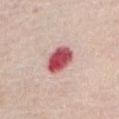No biopsy was performed on this lesion — it was imaged during a full skin examination and was not determined to be concerning. A region of skin cropped from a whole-body photographic capture, roughly 15 mm wide. The tile uses white-light illumination. Measured at roughly 4 mm in maximum diameter. The lesion is on the abdomen. A female patient approximately 65 years of age.